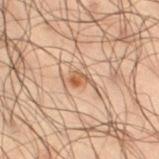Q: Is there a histopathology result?
A: catalogued during a skin exam; not biopsied
Q: What are the patient's age and sex?
A: male, aged 48–52
Q: Where on the body is the lesion?
A: the right thigh
Q: Illumination type?
A: cross-polarized
Q: What is the lesion's diameter?
A: ≈2.5 mm
Q: What did automated image analysis measure?
A: about 9 CIELAB-L* units darker than the surrounding skin and a normalized lesion–skin contrast near 7.5
Q: What kind of image is this?
A: ~15 mm crop, total-body skin-cancer survey